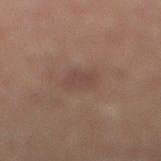| field | value |
|---|---|
| workup | no biopsy performed (imaged during a skin exam) |
| patient | male, roughly 60 years of age |
| lesion diameter | ≈3 mm |
| tile lighting | cross-polarized illumination |
| image | ~15 mm tile from a whole-body skin photo |
| anatomic site | the right lower leg |